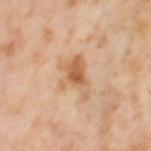{
  "biopsy_status": "not biopsied; imaged during a skin examination",
  "image": {
    "source": "total-body photography crop",
    "field_of_view_mm": 15
  },
  "lesion_size": {
    "long_diameter_mm_approx": 5.0
  },
  "patient": {
    "sex": "female",
    "age_approx": 55
  },
  "site": "left thigh"
}